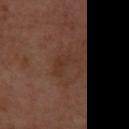Q: Was a biopsy performed?
A: total-body-photography surveillance lesion; no biopsy
Q: How was the tile lit?
A: cross-polarized illumination
Q: How was this image acquired?
A: total-body-photography crop, ~15 mm field of view
Q: Automated lesion metrics?
A: a lesion color around L≈34 a*≈20 b*≈27 in CIELAB, about 4 CIELAB-L* units darker than the surrounding skin, and a normalized border contrast of about 4.5; a classifier nevus-likeness of about 0/100 and a detector confidence of about 100 out of 100 that the crop contains a lesion
Q: Where on the body is the lesion?
A: the right forearm
Q: What is the lesion's diameter?
A: about 3.5 mm
Q: What are the patient's age and sex?
A: female, aged 48 to 52